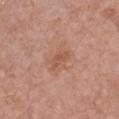Q: Was this lesion biopsied?
A: total-body-photography surveillance lesion; no biopsy
Q: What are the patient's age and sex?
A: female, aged 38 to 42
Q: How large is the lesion?
A: about 2.5 mm
Q: What is the imaging modality?
A: ~15 mm crop, total-body skin-cancer survey
Q: Illumination type?
A: white-light
Q: What did automated image analysis measure?
A: a nevus-likeness score of about 0/100 and a detector confidence of about 100 out of 100 that the crop contains a lesion
Q: What is the anatomic site?
A: the chest